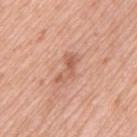Impression:
The lesion was tiled from a total-body skin photograph and was not biopsied.
Image and clinical context:
Captured under white-light illumination. Automated tile analysis of the lesion measured a lesion area of about 4.5 mm², an outline eccentricity of about 0.9 (0 = round, 1 = elongated), and a shape-asymmetry score of about 0.5 (0 = symmetric). The analysis additionally found a mean CIELAB color near L≈60 a*≈24 b*≈32, about 9 CIELAB-L* units darker than the surrounding skin, and a lesion-to-skin contrast of about 6 (normalized; higher = more distinct). It also reported a color-variation rating of about 2/10 and a peripheral color-asymmetry measure near 0.5. The patient is a female aged 68 to 72. From the left thigh. A close-up tile cropped from a whole-body skin photograph, about 15 mm across.